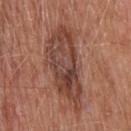The lesion was tiled from a total-body skin photograph and was not biopsied.
The lesion is on the head or neck.
The lesion's longest dimension is about 10 mm.
A 15 mm close-up extracted from a 3D total-body photography capture.
Automated tile analysis of the lesion measured a lesion area of about 28 mm² and a shape eccentricity near 0.9. The analysis additionally found border irregularity of about 5 on a 0–10 scale and internal color variation of about 7 on a 0–10 scale. The analysis additionally found a nevus-likeness score of about 25/100 and lesion-presence confidence of about 100/100.
The subject is a male about 80 years old.
Imaged with white-light lighting.